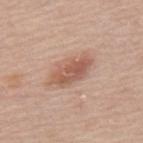Assessment: Recorded during total-body skin imaging; not selected for excision or biopsy. Acquisition and patient details: The subject is a female in their mid- to late 60s. An algorithmic analysis of the crop reported a border-irregularity rating of about 3/10. Captured under white-light illumination. The lesion is located on the mid back. A lesion tile, about 15 mm wide, cut from a 3D total-body photograph.